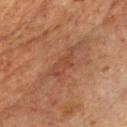Recorded during total-body skin imaging; not selected for excision or biopsy. A 15 mm close-up tile from a total-body photography series done for melanoma screening. The lesion is located on the chest. Automated image analysis of the tile measured an outline eccentricity of about 0.9 (0 = round, 1 = elongated) and a shape-asymmetry score of about 0.4 (0 = symmetric). The software also gave an average lesion color of about L≈36 a*≈20 b*≈26 (CIELAB), roughly 5 lightness units darker than nearby skin, and a normalized lesion–skin contrast near 5. The patient is a male in their mid- to late 60s. Measured at roughly 4 mm in maximum diameter.